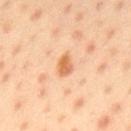No biopsy was performed on this lesion — it was imaged during a full skin examination and was not determined to be concerning.
A male patient in their mid-40s.
The lesion is located on the upper back.
Cropped from a total-body skin-imaging series; the visible field is about 15 mm.